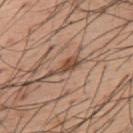| key | value |
|---|---|
| follow-up | imaged on a skin check; not biopsied |
| imaging modality | ~15 mm crop, total-body skin-cancer survey |
| anatomic site | the back |
| patient | male, roughly 55 years of age |